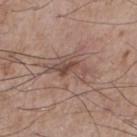Recorded during total-body skin imaging; not selected for excision or biopsy. The lesion is on the front of the torso. A male patient approximately 75 years of age. The tile uses white-light illumination. A 15 mm crop from a total-body photograph taken for skin-cancer surveillance. Measured at roughly 5 mm in maximum diameter.